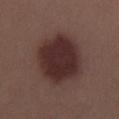The lesion was tiled from a total-body skin photograph and was not biopsied. An algorithmic analysis of the crop reported a lesion area of about 29 mm² and a shape-asymmetry score of about 0.1 (0 = symmetric). And it measured a mean CIELAB color near L≈29 a*≈19 b*≈18, a lesion–skin lightness drop of about 12, and a normalized lesion–skin contrast near 11.5. The patient is a female about 40 years old. Longest diameter approximately 6.5 mm. From the left thigh. The tile uses white-light illumination. A 15 mm close-up tile from a total-body photography series done for melanoma screening.